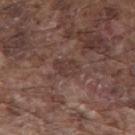Captured during whole-body skin photography for melanoma surveillance; the lesion was not biopsied. This is a white-light tile. A 15 mm close-up tile from a total-body photography series done for melanoma screening. On the back. A male patient, about 75 years old.